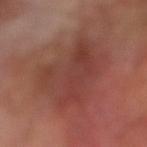{
  "biopsy_status": "not biopsied; imaged during a skin examination",
  "image": {
    "source": "total-body photography crop",
    "field_of_view_mm": 15
  },
  "lesion_size": {
    "long_diameter_mm_approx": 8.0
  },
  "patient": {
    "sex": "male",
    "age_approx": 70
  },
  "site": "right lower leg"
}